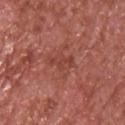{
  "biopsy_status": "not biopsied; imaged during a skin examination",
  "image": {
    "source": "total-body photography crop",
    "field_of_view_mm": 15
  },
  "lighting": "white-light",
  "automated_metrics": {
    "area_mm2_approx": 4.0,
    "cielab_L": 43,
    "cielab_a": 29,
    "cielab_b": 28,
    "vs_skin_contrast_norm": 5.0
  },
  "patient": {
    "sex": "male",
    "age_approx": 65
  },
  "site": "front of the torso"
}